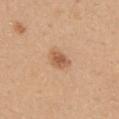workup: catalogued during a skin exam; not biopsied
illumination: white-light illumination
imaging modality: 15 mm crop, total-body photography
site: the mid back
size: about 3 mm
patient: male, about 40 years old